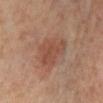Notes:
• notes: imaged on a skin check; not biopsied
• patient: female, about 55 years old
• body site: the right lower leg
• image: ~15 mm crop, total-body skin-cancer survey
• size: ≈4.5 mm
• tile lighting: cross-polarized